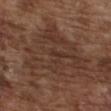follow-up: total-body-photography surveillance lesion; no biopsy
anatomic site: the chest
image source: 15 mm crop, total-body photography
size: ≈9.5 mm
subject: male, aged 73–77
lighting: white-light